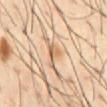Imaged during a routine full-body skin examination; the lesion was not biopsied and no histopathology is available. This image is a 15 mm lesion crop taken from a total-body photograph. Captured under cross-polarized illumination. From the chest. A male patient, aged around 40.